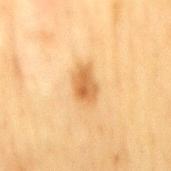| key | value |
|---|---|
| biopsy status | total-body-photography surveillance lesion; no biopsy |
| body site | the mid back |
| lighting | cross-polarized illumination |
| size | about 3.5 mm |
| patient | female, about 55 years old |
| imaging modality | total-body-photography crop, ~15 mm field of view |